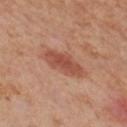A 15 mm close-up extracted from a 3D total-body photography capture. The recorded lesion diameter is about 5 mm. Captured under cross-polarized illumination. A female patient roughly 55 years of age. The lesion is located on the left thigh.